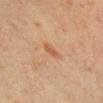{
  "patient": {
    "sex": "female",
    "age_approx": 40
  },
  "site": "chest",
  "lighting": "cross-polarized",
  "automated_metrics": {
    "eccentricity": 0.9,
    "border_irregularity_0_10": 2.5,
    "color_variation_0_10": 1.0
  },
  "image": {
    "source": "total-body photography crop",
    "field_of_view_mm": 15
  },
  "lesion_size": {
    "long_diameter_mm_approx": 3.0
  }
}